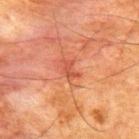<tbp_lesion>
<biopsy_status>not biopsied; imaged during a skin examination</biopsy_status>
<site>upper back</site>
<automated_metrics>
  <area_mm2_approx>3.0</area_mm2_approx>
  <shape_asymmetry>0.45</shape_asymmetry>
  <vs_skin_darker_L>7.0</vs_skin_darker_L>
  <vs_skin_contrast_norm>5.5</vs_skin_contrast_norm>
  <nevus_likeness_0_100>0</nevus_likeness_0_100>
</automated_metrics>
<patient>
  <sex>male</sex>
  <age_approx>65</age_approx>
</patient>
<lighting>cross-polarized</lighting>
<image>
  <source>total-body photography crop</source>
  <field_of_view_mm>15</field_of_view_mm>
</image>
<lesion_size>
  <long_diameter_mm_approx>2.5</long_diameter_mm_approx>
</lesion_size>
</tbp_lesion>